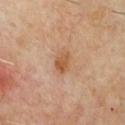• notes: no biopsy performed (imaged during a skin exam)
• tile lighting: cross-polarized
• location: the chest
• patient: male, in their mid-60s
• lesion size: ≈3 mm
• imaging modality: 15 mm crop, total-body photography